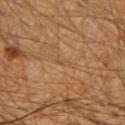Image and clinical context: From the right forearm. The recorded lesion diameter is about 1.5 mm. An algorithmic analysis of the crop reported an area of roughly 1 mm², an outline eccentricity of about 0.9 (0 = round, 1 = elongated), and two-axis asymmetry of about 0.35. Captured under cross-polarized illumination. A male patient, aged around 35. A 15 mm close-up tile from a total-body photography series done for melanoma screening.